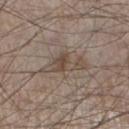Captured during whole-body skin photography for melanoma surveillance; the lesion was not biopsied. A 15 mm close-up extracted from a 3D total-body photography capture. This is a white-light tile. From the left lower leg. Measured at roughly 5.5 mm in maximum diameter. A male patient aged around 60.